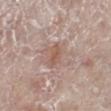This lesion was catalogued during total-body skin photography and was not selected for biopsy.
Approximately 2.5 mm at its widest.
Cropped from a total-body skin-imaging series; the visible field is about 15 mm.
Located on the leg.
Captured under white-light illumination.
The total-body-photography lesion software estimated a lesion area of about 2.5 mm² and an outline eccentricity of about 0.9 (0 = round, 1 = elongated). And it measured internal color variation of about 0.5 on a 0–10 scale and radial color variation of about 0. The software also gave an automated nevus-likeness rating near 55 out of 100.
A female patient aged 68–72.